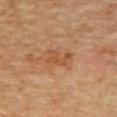{
  "biopsy_status": "not biopsied; imaged during a skin examination",
  "patient": {
    "sex": "female"
  },
  "image": {
    "source": "total-body photography crop",
    "field_of_view_mm": 15
  },
  "site": "upper back",
  "lesion_size": {
    "long_diameter_mm_approx": 3.0
  }
}